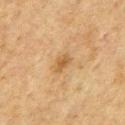Case summary:
* follow-up: no biopsy performed (imaged during a skin exam)
* illumination: cross-polarized illumination
* automated lesion analysis: a normalized border contrast of about 6.5; a border-irregularity rating of about 2.5/10 and internal color variation of about 2.5 on a 0–10 scale; a classifier nevus-likeness of about 10/100 and lesion-presence confidence of about 100/100
* image source: total-body-photography crop, ~15 mm field of view
* patient: male, aged 73–77
* location: the chest
* lesion diameter: ~2.5 mm (longest diameter)